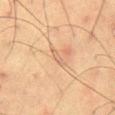Clinical impression:
The lesion was tiled from a total-body skin photograph and was not biopsied.
Image and clinical context:
Captured under cross-polarized illumination. A male patient, aged around 60. A 15 mm close-up extracted from a 3D total-body photography capture. Automated tile analysis of the lesion measured a lesion area of about 2 mm², a shape eccentricity near 0.95, and a shape-asymmetry score of about 0.35 (0 = symmetric). The analysis additionally found border irregularity of about 3.5 on a 0–10 scale, a color-variation rating of about 0/10, and a peripheral color-asymmetry measure near 0.